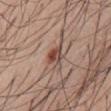Acquisition and patient details: An algorithmic analysis of the crop reported about 12 CIELAB-L* units darker than the surrounding skin and a normalized border contrast of about 9. From the abdomen. A roughly 15 mm field-of-view crop from a total-body skin photograph. Imaged with white-light lighting. The lesion's longest dimension is about 2.5 mm. A male patient aged 53 to 57.